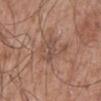| feature | finding |
|---|---|
| follow-up | catalogued during a skin exam; not biopsied |
| imaging modality | ~15 mm crop, total-body skin-cancer survey |
| body site | the mid back |
| patient | male, in their mid-50s |
| diameter | ~3 mm (longest diameter) |
| lighting | white-light |
| TBP lesion metrics | a lesion color around L≈49 a*≈18 b*≈25 in CIELAB, roughly 7 lightness units darker than nearby skin, and a normalized border contrast of about 5.5; a lesion-detection confidence of about 90/100 |